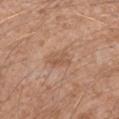Impression:
Captured during whole-body skin photography for melanoma surveillance; the lesion was not biopsied.
Image and clinical context:
The subject is a male in their 30s. A 15 mm close-up extracted from a 3D total-body photography capture. The lesion is on the left forearm.